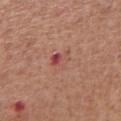Q: What are the patient's age and sex?
A: male, aged 63 to 67
Q: What is the imaging modality?
A: total-body-photography crop, ~15 mm field of view
Q: Lesion size?
A: ≈3 mm
Q: What did automated image analysis measure?
A: a lesion area of about 5.5 mm² and a shape-asymmetry score of about 0.35 (0 = symmetric); a mean CIELAB color near L≈51 a*≈26 b*≈26, roughly 7 lightness units darker than nearby skin, and a normalized lesion–skin contrast near 5.5; a color-variation rating of about 10/10 and peripheral color asymmetry of about 4
Q: What is the anatomic site?
A: the chest